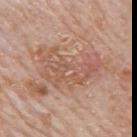This lesion was catalogued during total-body skin photography and was not selected for biopsy.
A region of skin cropped from a whole-body photographic capture, roughly 15 mm wide.
The lesion is on the mid back.
This is a white-light tile.
Automated tile analysis of the lesion measured an eccentricity of roughly 0.8 and a symmetry-axis asymmetry near 0.5. It also reported a border-irregularity index near 6.5/10 and a peripheral color-asymmetry measure near 1.5. The software also gave an automated nevus-likeness rating near 0 out of 100 and a lesion-detection confidence of about 100/100.
A male subject about 80 years old.
Approximately 7.5 mm at its widest.